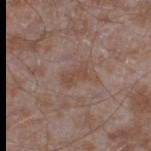Q: Was this lesion biopsied?
A: catalogued during a skin exam; not biopsied
Q: What are the patient's age and sex?
A: male, about 45 years old
Q: Where on the body is the lesion?
A: the right thigh
Q: Automated lesion metrics?
A: a mean CIELAB color near L≈46 a*≈17 b*≈25, roughly 6 lightness units darker than nearby skin, and a normalized lesion–skin contrast near 5.5; a border-irregularity index near 3.5/10, a color-variation rating of about 1.5/10, and peripheral color asymmetry of about 0.5; a classifier nevus-likeness of about 0/100 and a detector confidence of about 100 out of 100 that the crop contains a lesion
Q: What is the lesion's diameter?
A: ≈3.5 mm
Q: How was this image acquired?
A: ~15 mm tile from a whole-body skin photo
Q: How was the tile lit?
A: white-light